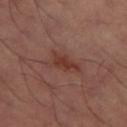Q: Is there a histopathology result?
A: no biopsy performed (imaged during a skin exam)
Q: How large is the lesion?
A: about 4.5 mm
Q: What lighting was used for the tile?
A: cross-polarized
Q: Lesion location?
A: the left thigh
Q: What kind of image is this?
A: ~15 mm tile from a whole-body skin photo